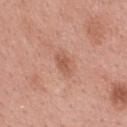Captured during whole-body skin photography for melanoma surveillance; the lesion was not biopsied.